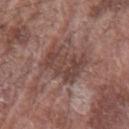{
  "biopsy_status": "not biopsied; imaged during a skin examination",
  "image": {
    "source": "total-body photography crop",
    "field_of_view_mm": 15
  },
  "automated_metrics": {
    "vs_skin_darker_L": 8.0,
    "vs_skin_contrast_norm": 6.5,
    "border_irregularity_0_10": 5.5,
    "color_variation_0_10": 3.0
  },
  "lighting": "white-light",
  "lesion_size": {
    "long_diameter_mm_approx": 4.5
  },
  "site": "left forearm",
  "patient": {
    "sex": "male",
    "age_approx": 75
  }
}